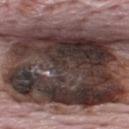– follow-up · catalogued during a skin exam; not biopsied
– site · the upper back
– size · ~17 mm (longest diameter)
– patient · male, about 70 years old
– acquisition · ~15 mm crop, total-body skin-cancer survey
– illumination · white-light illumination